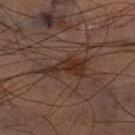Part of a total-body skin-imaging series; this lesion was reviewed on a skin check and was not flagged for biopsy. Measured at roughly 5.5 mm in maximum diameter. Automated image analysis of the tile measured an area of roughly 9 mm² and a symmetry-axis asymmetry near 0.45. The analysis additionally found a mean CIELAB color near L≈31 a*≈18 b*≈24. The analysis additionally found a border-irregularity index near 6/10, a color-variation rating of about 4/10, and a peripheral color-asymmetry measure near 1. The analysis additionally found a classifier nevus-likeness of about 80/100 and lesion-presence confidence of about 100/100. Cropped from a whole-body photographic skin survey; the tile spans about 15 mm. This is a cross-polarized tile. From the right thigh.